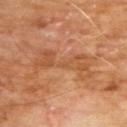Assessment: The lesion was tiled from a total-body skin photograph and was not biopsied. Acquisition and patient details: This is a cross-polarized tile. Located on the chest. A male patient, aged around 60. Approximately 7.5 mm at its widest. A roughly 15 mm field-of-view crop from a total-body skin photograph.